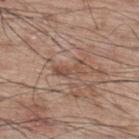The lesion was tiled from a total-body skin photograph and was not biopsied. Captured under white-light illumination. Automated image analysis of the tile measured an average lesion color of about L≈49 a*≈19 b*≈27 (CIELAB), roughly 8 lightness units darker than nearby skin, and a normalized border contrast of about 6. About 3.5 mm across. From the back. A male subject aged 68–72. A close-up tile cropped from a whole-body skin photograph, about 15 mm across.